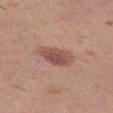Clinical impression:
Captured during whole-body skin photography for melanoma surveillance; the lesion was not biopsied.
Acquisition and patient details:
Located on the left thigh. The subject is a female in their 40s. A lesion tile, about 15 mm wide, cut from a 3D total-body photograph. Measured at roughly 4.5 mm in maximum diameter. This is a white-light tile.